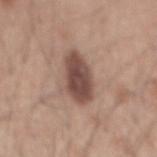Findings:
– notes — imaged on a skin check; not biopsied
– anatomic site — the mid back
– image source — ~15 mm crop, total-body skin-cancer survey
– patient — male, roughly 45 years of age